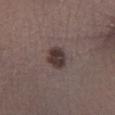Clinical impression: Captured during whole-body skin photography for melanoma surveillance; the lesion was not biopsied. Image and clinical context: Cropped from a whole-body photographic skin survey; the tile spans about 15 mm. A male subject, in their 40s. On the leg.